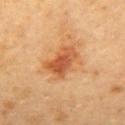Assessment: Imaged during a routine full-body skin examination; the lesion was not biopsied and no histopathology is available. Background: The lesion is located on the back. An algorithmic analysis of the crop reported a footprint of about 9.5 mm², an eccentricity of roughly 0.8, and a symmetry-axis asymmetry near 0.25. The software also gave a border-irregularity rating of about 3/10, a within-lesion color-variation index near 4.5/10, and radial color variation of about 1.5. The software also gave a nevus-likeness score of about 85/100 and lesion-presence confidence of about 100/100. The tile uses cross-polarized illumination. A 15 mm close-up tile from a total-body photography series done for melanoma screening. A female subject aged approximately 60.